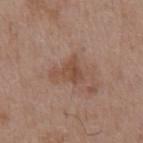The lesion was photographed on a routine skin check and not biopsied; there is no pathology result. The subject is a male in their mid-50s. The lesion is located on the abdomen. An algorithmic analysis of the crop reported a border-irregularity index near 7/10, a within-lesion color-variation index near 2.5/10, and radial color variation of about 1. The analysis additionally found a lesion-detection confidence of about 100/100. A 15 mm close-up tile from a total-body photography series done for melanoma screening. Approximately 3.5 mm at its widest.